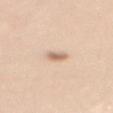  biopsy_status: not biopsied; imaged during a skin examination
  lighting: white-light
  automated_metrics:
    cielab_L: 67
    cielab_a: 18
    cielab_b: 31
    vs_skin_contrast_norm: 7.0
    border_irregularity_0_10: 3.0
    color_variation_0_10: 2.0
    nevus_likeness_0_100: 95
    lesion_detection_confidence_0_100: 100
  site: mid back
  lesion_size:
    long_diameter_mm_approx: 2.5
  image:
    source: total-body photography crop
    field_of_view_mm: 15
  patient:
    sex: female
    age_approx: 40